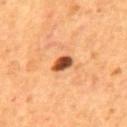<record>
  <biopsy_status>not biopsied; imaged during a skin examination</biopsy_status>
  <site>mid back</site>
  <image>
    <source>total-body photography crop</source>
    <field_of_view_mm>15</field_of_view_mm>
  </image>
  <lesion_size>
    <long_diameter_mm_approx>3.0</long_diameter_mm_approx>
  </lesion_size>
  <lighting>cross-polarized</lighting>
  <patient>
    <sex>male</sex>
    <age_approx>55</age_approx>
  </patient>
  <automated_metrics>
    <area_mm2_approx>4.0</area_mm2_approx>
    <eccentricity>0.8</eccentricity>
    <shape_asymmetry>0.15</shape_asymmetry>
    <border_irregularity_0_10>1.5</border_irregularity_0_10>
    <color_variation_0_10>5.0</color_variation_0_10>
    <peripheral_color_asymmetry>1.5</peripheral_color_asymmetry>
    <nevus_likeness_0_100>95</nevus_likeness_0_100>
    <lesion_detection_confidence_0_100>100</lesion_detection_confidence_0_100>
  </automated_metrics>
</record>